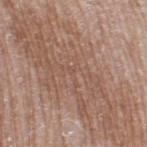Located on the leg.
This is a white-light tile.
Cropped from a total-body skin-imaging series; the visible field is about 15 mm.
The lesion-visualizer software estimated an area of roughly 100 mm², a shape eccentricity near 0.95, and a symmetry-axis asymmetry near 0.2. And it measured a classifier nevus-likeness of about 0/100 and lesion-presence confidence of about 60/100.
A male subject, roughly 65 years of age.